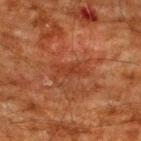Part of a total-body skin-imaging series; this lesion was reviewed on a skin check and was not flagged for biopsy.
A 15 mm close-up extracted from a 3D total-body photography capture.
The patient is a male roughly 60 years of age.
An algorithmic analysis of the crop reported roughly 5 lightness units darker than nearby skin and a lesion-to-skin contrast of about 5 (normalized; higher = more distinct). The analysis additionally found a border-irregularity rating of about 3.5/10, a color-variation rating of about 0/10, and a peripheral color-asymmetry measure near 0.
Imaged with cross-polarized lighting.
Located on the upper back.
The recorded lesion diameter is about 3 mm.